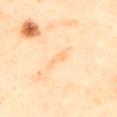Q: Was this lesion biopsied?
A: no biopsy performed (imaged during a skin exam)
Q: What lighting was used for the tile?
A: cross-polarized
Q: Who is the patient?
A: male, aged around 65
Q: How large is the lesion?
A: ≈4 mm
Q: What is the imaging modality?
A: total-body-photography crop, ~15 mm field of view
Q: Where on the body is the lesion?
A: the back